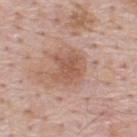<lesion>
  <biopsy_status>not biopsied; imaged during a skin examination</biopsy_status>
  <lesion_size>
    <long_diameter_mm_approx>4.0</long_diameter_mm_approx>
  </lesion_size>
  <image>
    <source>total-body photography crop</source>
    <field_of_view_mm>15</field_of_view_mm>
  </image>
  <patient>
    <sex>male</sex>
    <age_approx>50</age_approx>
  </patient>
  <site>upper back</site>
  <lighting>white-light</lighting>
</lesion>